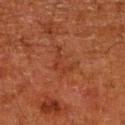biopsy_status: not biopsied; imaged during a skin examination
image:
  source: total-body photography crop
  field_of_view_mm: 15
lesion_size:
  long_diameter_mm_approx: 4.0
site: right lower leg
patient:
  sex: male
  age_approx: 80